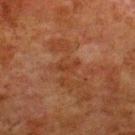Imaged during a routine full-body skin examination; the lesion was not biopsied and no histopathology is available.
This is a cross-polarized tile.
An algorithmic analysis of the crop reported a footprint of about 2 mm² and two-axis asymmetry of about 0.5.
The lesion's longest dimension is about 2.5 mm.
A male subject, aged 78–82.
Located on the upper back.
A region of skin cropped from a whole-body photographic capture, roughly 15 mm wide.